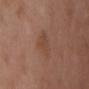follow-up = total-body-photography surveillance lesion; no biopsy | acquisition = 15 mm crop, total-body photography | location = the chest | lesion size = ≈3.5 mm | subject = female, about 60 years old.